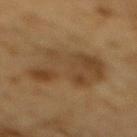Imaged during a routine full-body skin examination; the lesion was not biopsied and no histopathology is available. A male subject, roughly 85 years of age or older. From the mid back. A region of skin cropped from a whole-body photographic capture, roughly 15 mm wide.